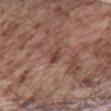Q: Is there a histopathology result?
A: catalogued during a skin exam; not biopsied
Q: Where on the body is the lesion?
A: the front of the torso
Q: What did automated image analysis measure?
A: a classifier nevus-likeness of about 0/100
Q: How was the tile lit?
A: white-light
Q: Lesion size?
A: ~2.5 mm (longest diameter)
Q: What is the imaging modality?
A: 15 mm crop, total-body photography
Q: Patient demographics?
A: male, approximately 75 years of age